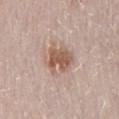This lesion was catalogued during total-body skin photography and was not selected for biopsy.
From the mid back.
The lesion-visualizer software estimated a classifier nevus-likeness of about 45/100 and a detector confidence of about 100 out of 100 that the crop contains a lesion.
A 15 mm close-up extracted from a 3D total-body photography capture.
The recorded lesion diameter is about 4 mm.
A female patient, aged around 30.
This is a white-light tile.